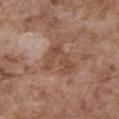{"biopsy_status": "not biopsied; imaged during a skin examination", "site": "front of the torso", "lighting": "white-light", "image": {"source": "total-body photography crop", "field_of_view_mm": 15}, "lesion_size": {"long_diameter_mm_approx": 4.5}, "patient": {"sex": "male", "age_approx": 75}}